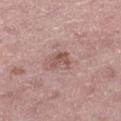This lesion was catalogued during total-body skin photography and was not selected for biopsy. Located on the left thigh. A 15 mm close-up extracted from a 3D total-body photography capture. Captured under white-light illumination. Approximately 2.5 mm at its widest. The total-body-photography lesion software estimated a footprint of about 4.5 mm², an eccentricity of roughly 0.55, and a symmetry-axis asymmetry near 0.35. The analysis additionally found an automated nevus-likeness rating near 25 out of 100 and a lesion-detection confidence of about 100/100. A female subject about 45 years old.